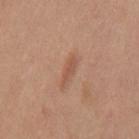workup: no biopsy performed (imaged during a skin exam)
tile lighting: white-light illumination
patient: male, about 30 years old
TBP lesion metrics: an area of roughly 3 mm², an outline eccentricity of about 0.9 (0 = round, 1 = elongated), and a symmetry-axis asymmetry near 0.4; a lesion–skin lightness drop of about 8 and a lesion-to-skin contrast of about 5.5 (normalized; higher = more distinct); a border-irregularity rating of about 4/10 and a color-variation rating of about 0.5/10
acquisition: total-body-photography crop, ~15 mm field of view
size: ~3 mm (longest diameter)
location: the mid back